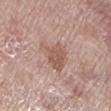Case summary:
• follow-up: catalogued during a skin exam; not biopsied
• subject: female, in their 70s
• location: the left lower leg
• imaging modality: ~15 mm crop, total-body skin-cancer survey